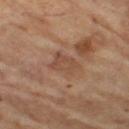Findings:
* lighting: cross-polarized
* lesion diameter: about 4 mm
* site: the left thigh
* image source: ~15 mm tile from a whole-body skin photo
* subject: female, aged approximately 70